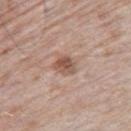Clinical impression: Part of a total-body skin-imaging series; this lesion was reviewed on a skin check and was not flagged for biopsy. Image and clinical context: Automated tile analysis of the lesion measured a mean CIELAB color near L≈53 a*≈19 b*≈27 and roughly 11 lightness units darker than nearby skin. The analysis additionally found a border-irregularity rating of about 2/10, a within-lesion color-variation index near 3.5/10, and radial color variation of about 1. It also reported an automated nevus-likeness rating near 10 out of 100. Located on the right thigh. The subject is a male aged 73 to 77. Cropped from a whole-body photographic skin survey; the tile spans about 15 mm. Imaged with white-light lighting.